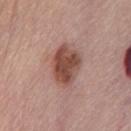notes: total-body-photography surveillance lesion; no biopsy
acquisition: ~15 mm crop, total-body skin-cancer survey
site: the front of the torso
tile lighting: white-light
patient: female, aged approximately 70
TBP lesion metrics: an outline eccentricity of about 0.7 (0 = round, 1 = elongated) and two-axis asymmetry of about 0.2; border irregularity of about 2 on a 0–10 scale, a color-variation rating of about 4/10, and peripheral color asymmetry of about 1.5; a nevus-likeness score of about 75/100 and a lesion-detection confidence of about 100/100